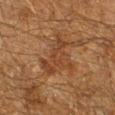The lesion is on the right lower leg.
A roughly 15 mm field-of-view crop from a total-body skin photograph.
The subject is a male about 60 years old.